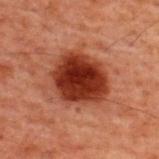TBP lesion metrics — a footprint of about 21 mm², an eccentricity of roughly 0.55, and a symmetry-axis asymmetry near 0.15; an average lesion color of about L≈26 a*≈26 b*≈27 (CIELAB) and a normalized border contrast of about 14 | patient — male, aged 58–62 | acquisition — ~15 mm crop, total-body skin-cancer survey | body site — the upper back.